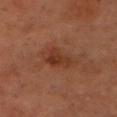Image and clinical context:
A lesion tile, about 15 mm wide, cut from a 3D total-body photograph. A male subject, approximately 55 years of age. On the head or neck.